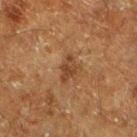No biopsy was performed on this lesion — it was imaged during a full skin examination and was not determined to be concerning. A male subject, aged around 60. A region of skin cropped from a whole-body photographic capture, roughly 15 mm wide. The lesion is on the leg. Captured under cross-polarized illumination. The recorded lesion diameter is about 3 mm.